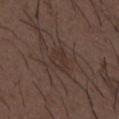• follow-up — total-body-photography surveillance lesion; no biopsy
• illumination — white-light illumination
• image-analysis metrics — an average lesion color of about L≈32 a*≈14 b*≈20 (CIELAB), roughly 4 lightness units darker than nearby skin, and a normalized border contrast of about 4.5; border irregularity of about 2 on a 0–10 scale, a color-variation rating of about 2/10, and radial color variation of about 0.5
• body site — the back
• image — ~15 mm tile from a whole-body skin photo
• patient — male, roughly 50 years of age
• lesion size — ≈3 mm